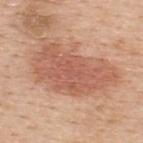The lesion was tiled from a total-body skin photograph and was not biopsied. The lesion is on the upper back. Cropped from a whole-body photographic skin survey; the tile spans about 15 mm. The patient is a male approximately 50 years of age.